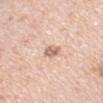<lesion>
  <biopsy_status>not biopsied; imaged during a skin examination</biopsy_status>
  <patient>
    <sex>female</sex>
    <age_approx>40</age_approx>
  </patient>
  <image>
    <source>total-body photography crop</source>
    <field_of_view_mm>15</field_of_view_mm>
  </image>
  <site>right upper arm</site>
</lesion>